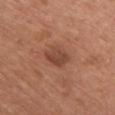The lesion was tiled from a total-body skin photograph and was not biopsied.
The lesion is on the chest.
The lesion's longest dimension is about 3.5 mm.
Cropped from a total-body skin-imaging series; the visible field is about 15 mm.
The subject is a female approximately 65 years of age.
Imaged with white-light lighting.
The total-body-photography lesion software estimated a lesion area of about 6 mm², a shape eccentricity near 0.7, and a shape-asymmetry score of about 0.3 (0 = symmetric). The software also gave about 9 CIELAB-L* units darker than the surrounding skin and a lesion-to-skin contrast of about 7 (normalized; higher = more distinct). And it measured a border-irregularity rating of about 3/10, a within-lesion color-variation index near 2.5/10, and a peripheral color-asymmetry measure near 1.